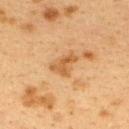Part of a total-body skin-imaging series; this lesion was reviewed on a skin check and was not flagged for biopsy. The total-body-photography lesion software estimated a lesion area of about 5.5 mm² and a symmetry-axis asymmetry near 0.5. It also reported an average lesion color of about L≈49 a*≈19 b*≈37 (CIELAB), roughly 8 lightness units darker than nearby skin, and a normalized lesion–skin contrast near 7. A lesion tile, about 15 mm wide, cut from a 3D total-body photograph. Approximately 3.5 mm at its widest. The tile uses cross-polarized illumination. The lesion is located on the upper back. A female subject, aged around 40.